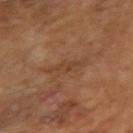Part of a total-body skin-imaging series; this lesion was reviewed on a skin check and was not flagged for biopsy. On the left arm. Automated tile analysis of the lesion measured an area of roughly 3.5 mm², an outline eccentricity of about 0.95 (0 = round, 1 = elongated), and a shape-asymmetry score of about 0.35 (0 = symmetric). The software also gave an average lesion color of about L≈39 a*≈19 b*≈31 (CIELAB), roughly 6 lightness units darker than nearby skin, and a lesion-to-skin contrast of about 5.5 (normalized; higher = more distinct). The analysis additionally found a border-irregularity index near 5.5/10, a within-lesion color-variation index near 0.5/10, and peripheral color asymmetry of about 0. Longest diameter approximately 4 mm. A 15 mm close-up tile from a total-body photography series done for melanoma screening. The patient is a male aged approximately 65.